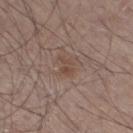Notes:
- TBP lesion metrics: a lesion area of about 2.5 mm², an eccentricity of roughly 0.9, and two-axis asymmetry of about 0.4
- patient: male, about 55 years old
- image source: ~15 mm tile from a whole-body skin photo
- lesion diameter: ≈2.5 mm
- lighting: white-light
- location: the left lower leg